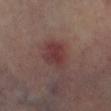A male patient, approximately 65 years of age. Captured under cross-polarized illumination. The lesion is on the left lower leg. Longest diameter approximately 4.5 mm. A lesion tile, about 15 mm wide, cut from a 3D total-body photograph.